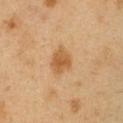Q: Was a biopsy performed?
A: catalogued during a skin exam; not biopsied
Q: What kind of image is this?
A: 15 mm crop, total-body photography
Q: Where on the body is the lesion?
A: the right upper arm
Q: Illumination type?
A: cross-polarized
Q: Lesion size?
A: about 3.5 mm
Q: Automated lesion metrics?
A: a lesion-detection confidence of about 100/100
Q: Who is the patient?
A: male, aged 48–52